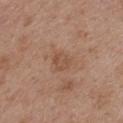Clinical summary:
A 15 mm close-up extracted from a 3D total-body photography capture. The subject is a female roughly 40 years of age. Located on the upper back.